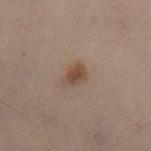patient = female, aged 48–52; image = ~15 mm tile from a whole-body skin photo; site = the leg; lighting = cross-polarized.